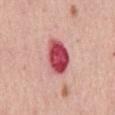Impression:
This lesion was catalogued during total-body skin photography and was not selected for biopsy.
Acquisition and patient details:
From the mid back. A female subject roughly 60 years of age. Automated tile analysis of the lesion measured a lesion color around L≈51 a*≈39 b*≈22 in CIELAB, about 20 CIELAB-L* units darker than the surrounding skin, and a normalized border contrast of about 13. And it measured a peripheral color-asymmetry measure near 2. A lesion tile, about 15 mm wide, cut from a 3D total-body photograph.